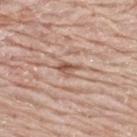Imaged during a routine full-body skin examination; the lesion was not biopsied and no histopathology is available. A male patient, aged 78 to 82. The tile uses white-light illumination. A 15 mm crop from a total-body photograph taken for skin-cancer surveillance. The lesion is located on the upper back. Approximately 3 mm at its widest. An algorithmic analysis of the crop reported a footprint of about 3.5 mm², a shape eccentricity near 0.75, and a shape-asymmetry score of about 0.4 (0 = symmetric). The software also gave border irregularity of about 4 on a 0–10 scale, internal color variation of about 5 on a 0–10 scale, and a peripheral color-asymmetry measure near 2.